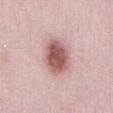notes=total-body-photography surveillance lesion; no biopsy